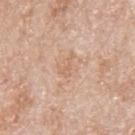notes: no biopsy performed (imaged during a skin exam) | automated metrics: a lesion area of about 2.5 mm² and an outline eccentricity of about 0.9 (0 = round, 1 = elongated); an average lesion color of about L≈64 a*≈19 b*≈32 (CIELAB), a lesion–skin lightness drop of about 6, and a normalized border contrast of about 4.5; a within-lesion color-variation index near 0/10 and a peripheral color-asymmetry measure near 0; a nevus-likeness score of about 0/100 and a lesion-detection confidence of about 100/100 | body site: the upper back | acquisition: 15 mm crop, total-body photography | illumination: white-light | size: ≈3 mm | subject: male, approximately 80 years of age.